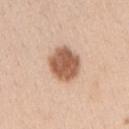biopsy status: no biopsy performed (imaged during a skin exam) | lesion diameter: ≈4 mm | subject: female, approximately 40 years of age | image source: 15 mm crop, total-body photography | anatomic site: the right upper arm | illumination: white-light.